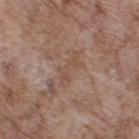Assessment: No biopsy was performed on this lesion — it was imaged during a full skin examination and was not determined to be concerning. Context: A 15 mm close-up extracted from a 3D total-body photography capture. Automated image analysis of the tile measured an outline eccentricity of about 0.95 (0 = round, 1 = elongated). And it measured a border-irregularity index near 8.5/10, internal color variation of about 1.5 on a 0–10 scale, and radial color variation of about 0. It also reported an automated nevus-likeness rating near 0 out of 100 and lesion-presence confidence of about 70/100. Located on the upper back. Imaged with white-light lighting. A male subject roughly 70 years of age.